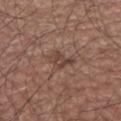Impression:
No biopsy was performed on this lesion — it was imaged during a full skin examination and was not determined to be concerning.
Acquisition and patient details:
About 3 mm across. The lesion is on the left lower leg. Cropped from a total-body skin-imaging series; the visible field is about 15 mm. A male patient about 75 years old. Imaged with white-light lighting. The lesion-visualizer software estimated an area of roughly 4.5 mm², an outline eccentricity of about 0.75 (0 = round, 1 = elongated), and a symmetry-axis asymmetry near 0.4. And it measured a lesion color around L≈42 a*≈18 b*≈25 in CIELAB, a lesion–skin lightness drop of about 8, and a lesion-to-skin contrast of about 6.5 (normalized; higher = more distinct).